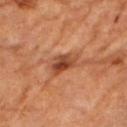This lesion was catalogued during total-body skin photography and was not selected for biopsy. An algorithmic analysis of the crop reported a footprint of about 7 mm², an outline eccentricity of about 0.85 (0 = round, 1 = elongated), and a shape-asymmetry score of about 0.25 (0 = symmetric). It also reported an average lesion color of about L≈45 a*≈26 b*≈34 (CIELAB), a lesion–skin lightness drop of about 12, and a normalized border contrast of about 8.5. The analysis additionally found a detector confidence of about 100 out of 100 that the crop contains a lesion. On the left arm. Captured under cross-polarized illumination. About 4 mm across. The patient is a male aged 58 to 62. A roughly 15 mm field-of-view crop from a total-body skin photograph.